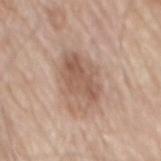Image and clinical context:
Cropped from a total-body skin-imaging series; the visible field is about 15 mm. The patient is a male roughly 80 years of age. The lesion is located on the mid back. Automated tile analysis of the lesion measured a footprint of about 16 mm², a shape eccentricity near 0.75, and a symmetry-axis asymmetry near 0.2. And it measured a mean CIELAB color near L≈57 a*≈18 b*≈27 and about 10 CIELAB-L* units darker than the surrounding skin. And it measured a within-lesion color-variation index near 5/10 and a peripheral color-asymmetry measure near 2. The analysis additionally found a nevus-likeness score of about 35/100. Measured at roughly 6 mm in maximum diameter. The tile uses white-light illumination.